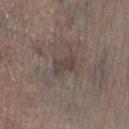{
  "biopsy_status": "not biopsied; imaged during a skin examination",
  "patient": {
    "sex": "male",
    "age_approx": 75
  },
  "lighting": "white-light",
  "site": "left lower leg",
  "image": {
    "source": "total-body photography crop",
    "field_of_view_mm": 15
  }
}